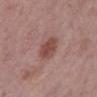About 3 mm across.
A male subject aged around 60.
A roughly 15 mm field-of-view crop from a total-body skin photograph.
The tile uses white-light illumination.
The lesion is on the back.